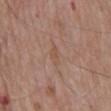Recorded during total-body skin imaging; not selected for excision or biopsy. A male patient, aged 78 to 82. Located on the back. Cropped from a total-body skin-imaging series; the visible field is about 15 mm. The total-body-photography lesion software estimated an average lesion color of about L≈52 a*≈19 b*≈28 (CIELAB), a lesion–skin lightness drop of about 5, and a normalized lesion–skin contrast near 4.5. It also reported an automated nevus-likeness rating near 0 out of 100 and lesion-presence confidence of about 90/100. About 3 mm across.